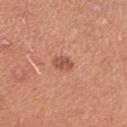Clinical impression: Recorded during total-body skin imaging; not selected for excision or biopsy. Background: A female patient approximately 25 years of age. The total-body-photography lesion software estimated a shape eccentricity near 0.75 and a symmetry-axis asymmetry near 0.2. The lesion's longest dimension is about 2.5 mm. The lesion is located on the right upper arm. A region of skin cropped from a whole-body photographic capture, roughly 15 mm wide.